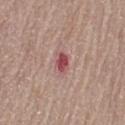Findings:
– biopsy status — no biopsy performed (imaged during a skin exam)
– automated metrics — a footprint of about 3.5 mm² and a symmetry-axis asymmetry near 0.25; an average lesion color of about L≈50 a*≈29 b*≈20 (CIELAB), roughly 13 lightness units darker than nearby skin, and a lesion-to-skin contrast of about 9.5 (normalized; higher = more distinct)
– imaging modality — 15 mm crop, total-body photography
– site — the leg
– lesion diameter — about 2.5 mm
– patient — female, aged 63 to 67
– tile lighting — white-light illumination